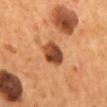Assessment: The lesion was tiled from a total-body skin photograph and was not biopsied. Acquisition and patient details: Automated tile analysis of the lesion measured an area of roughly 7.5 mm², an eccentricity of roughly 0.7, and a symmetry-axis asymmetry near 0.2. It also reported an average lesion color of about L≈43 a*≈24 b*≈33 (CIELAB). The analysis additionally found a border-irregularity rating of about 1.5/10, a color-variation rating of about 6.5/10, and peripheral color asymmetry of about 2.5. The analysis additionally found a detector confidence of about 100 out of 100 that the crop contains a lesion. On the mid back. This is a cross-polarized tile. The patient is a male approximately 55 years of age. This image is a 15 mm lesion crop taken from a total-body photograph.